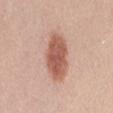Assessment:
No biopsy was performed on this lesion — it was imaged during a full skin examination and was not determined to be concerning.
Image and clinical context:
A 15 mm close-up tile from a total-body photography series done for melanoma screening. A female patient about 25 years old. The tile uses white-light illumination. The lesion-visualizer software estimated a footprint of about 16 mm², a shape eccentricity near 0.8, and a symmetry-axis asymmetry near 0.15. It also reported a classifier nevus-likeness of about 100/100. On the right thigh.